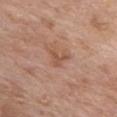notes = catalogued during a skin exam; not biopsied
subject = female, in their mid-70s
automated metrics = a footprint of about 3.5 mm², a shape eccentricity near 0.55, and two-axis asymmetry of about 0.5; border irregularity of about 5 on a 0–10 scale, a color-variation rating of about 0.5/10, and peripheral color asymmetry of about 0; a classifier nevus-likeness of about 0/100
imaging modality = ~15 mm tile from a whole-body skin photo
tile lighting = white-light illumination
lesion size = about 2.5 mm
location = the front of the torso